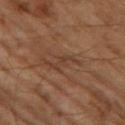follow-up: no biopsy performed (imaged during a skin exam) | subject: male, aged around 65 | lighting: cross-polarized illumination | image: total-body-photography crop, ~15 mm field of view.